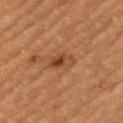  site: left upper arm
  image:
    source: total-body photography crop
    field_of_view_mm: 15
  patient:
    sex: female
    age_approx: 70